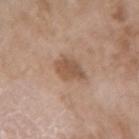biopsy status=no biopsy performed (imaged during a skin exam) | subject=female, aged 73–77 | anatomic site=the arm | lesion size=~3.5 mm (longest diameter) | imaging modality=total-body-photography crop, ~15 mm field of view | lighting=white-light illumination.